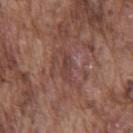biopsy status=total-body-photography surveillance lesion; no biopsy | tile lighting=white-light | site=the chest | subject=male, about 75 years old | lesion size=~3.5 mm (longest diameter) | image=total-body-photography crop, ~15 mm field of view | TBP lesion metrics=an area of roughly 3.5 mm² and two-axis asymmetry of about 0.4.